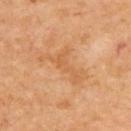The lesion was tiled from a total-body skin photograph and was not biopsied. This image is a 15 mm lesion crop taken from a total-body photograph. Approximately 6 mm at its widest. From the upper back. The subject is aged 63 to 67.